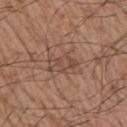Clinical impression: No biopsy was performed on this lesion — it was imaged during a full skin examination and was not determined to be concerning. Acquisition and patient details: On the arm. This is a white-light tile. A male patient about 55 years old. A 15 mm crop from a total-body photograph taken for skin-cancer surveillance. An algorithmic analysis of the crop reported a mean CIELAB color near L≈47 a*≈19 b*≈28, about 7 CIELAB-L* units darker than the surrounding skin, and a lesion-to-skin contrast of about 5.5 (normalized; higher = more distinct). The analysis additionally found a border-irregularity rating of about 5.5/10 and radial color variation of about 0.5.